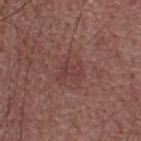The lesion was tiled from a total-body skin photograph and was not biopsied. A male patient aged 53 to 57. A 15 mm crop from a total-body photograph taken for skin-cancer surveillance. From the upper back. Longest diameter approximately 3 mm.